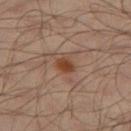| key | value |
|---|---|
| workup | no biopsy performed (imaged during a skin exam) |
| subject | male, in their mid-60s |
| lighting | cross-polarized illumination |
| site | the left thigh |
| diameter | about 2.5 mm |
| imaging modality | 15 mm crop, total-body photography |